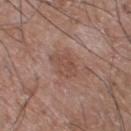image = ~15 mm tile from a whole-body skin photo
lighting = white-light illumination
location = the left lower leg
subject = male, aged around 60
lesion size = ~3.5 mm (longest diameter)